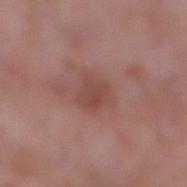Captured during whole-body skin photography for melanoma surveillance; the lesion was not biopsied. A female subject, aged approximately 70. Automated image analysis of the tile measured a footprint of about 6.5 mm², an outline eccentricity of about 0.5 (0 = round, 1 = elongated), and two-axis asymmetry of about 0.25. It also reported a within-lesion color-variation index near 2.5/10. A 15 mm crop from a total-body photograph taken for skin-cancer surveillance. The tile uses white-light illumination. The lesion is on the right lower leg. The lesion's longest dimension is about 3 mm.